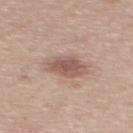workup = catalogued during a skin exam; not biopsied
image = total-body-photography crop, ~15 mm field of view
image-analysis metrics = an average lesion color of about L≈55 a*≈19 b*≈23 (CIELAB), about 11 CIELAB-L* units darker than the surrounding skin, and a normalized border contrast of about 7.5; a peripheral color-asymmetry measure near 1; a nevus-likeness score of about 55/100 and a detector confidence of about 100 out of 100 that the crop contains a lesion
site = the mid back
patient = male, roughly 55 years of age
illumination = white-light
lesion diameter = about 4 mm